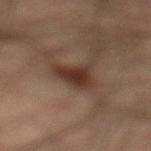| key | value |
|---|---|
| follow-up | no biopsy performed (imaged during a skin exam) |
| diameter | about 3.5 mm |
| site | the left lower leg |
| tile lighting | cross-polarized illumination |
| subject | male, in their mid- to late 30s |
| acquisition | 15 mm crop, total-body photography |